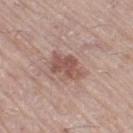The lesion was tiled from a total-body skin photograph and was not biopsied. A male subject aged 53 to 57. This image is a 15 mm lesion crop taken from a total-body photograph. Located on the right thigh. The tile uses white-light illumination. Approximately 4 mm at its widest. The total-body-photography lesion software estimated a footprint of about 8 mm², an outline eccentricity of about 0.75 (0 = round, 1 = elongated), and a shape-asymmetry score of about 0.35 (0 = symmetric). The analysis additionally found a mean CIELAB color near L≈52 a*≈21 b*≈24 and a normalized border contrast of about 7.5.